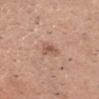Assessment:
This lesion was catalogued during total-body skin photography and was not selected for biopsy.
Context:
This is a white-light tile. The lesion's longest dimension is about 3.5 mm. The lesion is located on the head or neck. A male patient, aged 58–62. Cropped from a whole-body photographic skin survey; the tile spans about 15 mm.